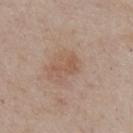{
  "biopsy_status": "not biopsied; imaged during a skin examination",
  "image": {
    "source": "total-body photography crop",
    "field_of_view_mm": 15
  },
  "lighting": "white-light",
  "site": "abdomen",
  "patient": {
    "sex": "male",
    "age_approx": 55
  },
  "automated_metrics": {
    "cielab_L": 54,
    "cielab_a": 19,
    "cielab_b": 29,
    "vs_skin_darker_L": 7.0,
    "vs_skin_contrast_norm": 5.5
  }
}